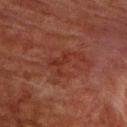  biopsy_status: not biopsied; imaged during a skin examination
  lesion_size:
    long_diameter_mm_approx: 4.5
  patient:
    sex: male
    age_approx: 60
  automated_metrics:
    border_irregularity_0_10: 9.5
    color_variation_0_10: 2.5
    peripheral_color_asymmetry: 1.0
    nevus_likeness_0_100: 0
    lesion_detection_confidence_0_100: 100
  image:
    source: total-body photography crop
    field_of_view_mm: 15
  site: front of the torso